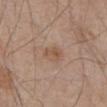biopsy_status: not biopsied; imaged during a skin examination
lesion_size:
  long_diameter_mm_approx: 2.5
site: abdomen
image:
  source: total-body photography crop
  field_of_view_mm: 15
lighting: white-light
patient:
  sex: male
  age_approx: 80